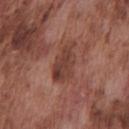Notes:
* follow-up: imaged on a skin check; not biopsied
* subject: male, roughly 75 years of age
* automated metrics: a lesion color around L≈40 a*≈23 b*≈25 in CIELAB, a lesion–skin lightness drop of about 9, and a normalized lesion–skin contrast near 7.5; an automated nevus-likeness rating near 0 out of 100
* body site: the chest
* tile lighting: white-light
* lesion size: ≈4.5 mm
* image source: ~15 mm crop, total-body skin-cancer survey